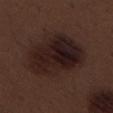Q: What is the anatomic site?
A: the left thigh
Q: What kind of image is this?
A: total-body-photography crop, ~15 mm field of view
Q: What did automated image analysis measure?
A: a border-irregularity rating of about 2/10 and a peripheral color-asymmetry measure near 2.5; a nevus-likeness score of about 60/100 and a detector confidence of about 100 out of 100 that the crop contains a lesion
Q: Illumination type?
A: white-light illumination
Q: What are the patient's age and sex?
A: male, approximately 70 years of age